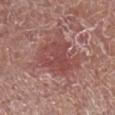Notes:
- biopsy status — total-body-photography surveillance lesion; no biopsy
- patient — male, approximately 55 years of age
- tile lighting — white-light
- image — total-body-photography crop, ~15 mm field of view
- location — the right lower leg
- lesion diameter — about 6 mm